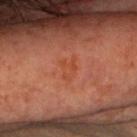Captured during whole-body skin photography for melanoma surveillance; the lesion was not biopsied.
A 15 mm close-up extracted from a 3D total-body photography capture.
Automated image analysis of the tile measured roughly 4 lightness units darker than nearby skin and a lesion-to-skin contrast of about 4.5 (normalized; higher = more distinct).
Captured under cross-polarized illumination.
The subject is a male aged 68–72.
Measured at roughly 3 mm in maximum diameter.
The lesion is located on the head or neck.